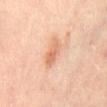The lesion was photographed on a routine skin check and not biopsied; there is no pathology result. A female patient approximately 50 years of age. A roughly 15 mm field-of-view crop from a total-body skin photograph. Located on the abdomen. Longest diameter approximately 3.5 mm. Imaged with cross-polarized lighting.